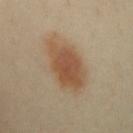image = 15 mm crop, total-body photography; body site = the mid back; diameter = ~7.5 mm (longest diameter); automated metrics = a color-variation rating of about 3.5/10 and radial color variation of about 1; patient = female, about 40 years old.